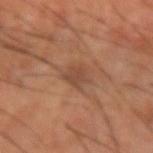– follow-up — no biopsy performed (imaged during a skin exam)
– TBP lesion metrics — a mean CIELAB color near L≈44 a*≈21 b*≈29, a lesion–skin lightness drop of about 7, and a lesion-to-skin contrast of about 6 (normalized; higher = more distinct)
– site — the right forearm
– image source — ~15 mm crop, total-body skin-cancer survey
– lesion size — ~2.5 mm (longest diameter)
– subject — male, about 60 years old